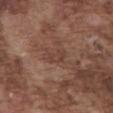Q: Was a biopsy performed?
A: catalogued during a skin exam; not biopsied
Q: Patient demographics?
A: male, aged approximately 75
Q: What is the anatomic site?
A: the abdomen
Q: What lighting was used for the tile?
A: white-light illumination
Q: How was this image acquired?
A: ~15 mm crop, total-body skin-cancer survey
Q: What did automated image analysis measure?
A: an area of roughly 4.5 mm², an eccentricity of roughly 0.8, and a symmetry-axis asymmetry near 0.4; a lesion color around L≈41 a*≈19 b*≈25 in CIELAB, roughly 6 lightness units darker than nearby skin, and a lesion-to-skin contrast of about 5 (normalized; higher = more distinct); a border-irregularity index near 4/10, a color-variation rating of about 2/10, and a peripheral color-asymmetry measure near 0.5; a nevus-likeness score of about 0/100 and a lesion-detection confidence of about 95/100
Q: What is the lesion's diameter?
A: ≈3 mm